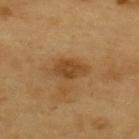Longest diameter approximately 4 mm.
On the upper back.
The patient is a male aged around 60.
A 15 mm crop from a total-body photograph taken for skin-cancer surveillance.